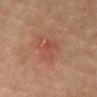Notes:
• biopsy status: catalogued during a skin exam; not biopsied
• size: ≈4.5 mm
• patient: male, roughly 75 years of age
• anatomic site: the front of the torso
• image-analysis metrics: a footprint of about 9.5 mm², an outline eccentricity of about 0.7 (0 = round, 1 = elongated), and two-axis asymmetry of about 0.35; a lesion color around L≈40 a*≈22 b*≈24 in CIELAB and a normalized border contrast of about 4.5; a border-irregularity rating of about 5/10, a color-variation rating of about 2.5/10, and radial color variation of about 1
• image source: ~15 mm crop, total-body skin-cancer survey
• illumination: cross-polarized